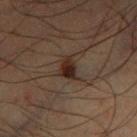Findings:
- patient: male, about 50 years old
- body site: the right lower leg
- diameter: about 2.5 mm
- acquisition: ~15 mm tile from a whole-body skin photo
- automated metrics: an eccentricity of roughly 0.75 and a symmetry-axis asymmetry near 0.35; about 9 CIELAB-L* units darker than the surrounding skin and a lesion-to-skin contrast of about 11 (normalized; higher = more distinct); a classifier nevus-likeness of about 95/100 and a lesion-detection confidence of about 100/100